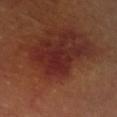Impression:
This lesion was catalogued during total-body skin photography and was not selected for biopsy.
Clinical summary:
An algorithmic analysis of the crop reported internal color variation of about 2 on a 0–10 scale and peripheral color asymmetry of about 0.5. A close-up tile cropped from a whole-body skin photograph, about 15 mm across. On the right upper arm. A male patient approximately 65 years of age. Imaged with cross-polarized lighting. Approximately 4 mm at its widest.